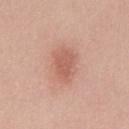* biopsy status: imaged on a skin check; not biopsied
* body site: the abdomen
* diameter: ≈3.5 mm
* patient: male, about 40 years old
* imaging modality: 15 mm crop, total-body photography